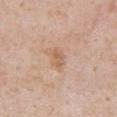<tbp_lesion>
<biopsy_status>not biopsied; imaged during a skin examination</biopsy_status>
<lighting>white-light</lighting>
<lesion_size>
  <long_diameter_mm_approx>2.5</long_diameter_mm_approx>
</lesion_size>
<automated_metrics>
  <area_mm2_approx>3.0</area_mm2_approx>
  <eccentricity>0.85</eccentricity>
  <shape_asymmetry>0.3</shape_asymmetry>
  <cielab_L>61</cielab_L>
  <cielab_a>19</cielab_a>
  <cielab_b>33</cielab_b>
  <vs_skin_darker_L>8.0</vs_skin_darker_L>
  <vs_skin_contrast_norm>6.0</vs_skin_contrast_norm>
  <nevus_likeness_0_100>5</nevus_likeness_0_100>
  <lesion_detection_confidence_0_100>100</lesion_detection_confidence_0_100>
</automated_metrics>
<image>
  <source>total-body photography crop</source>
  <field_of_view_mm>15</field_of_view_mm>
</image>
<patient>
  <sex>male</sex>
  <age_approx>65</age_approx>
</patient>
<site>chest</site>
</tbp_lesion>